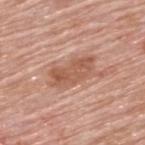Impression:
Imaged during a routine full-body skin examination; the lesion was not biopsied and no histopathology is available.
Background:
About 5.5 mm across. This is a white-light tile. A lesion tile, about 15 mm wide, cut from a 3D total-body photograph. Automated image analysis of the tile measured a footprint of about 11 mm² and an outline eccentricity of about 0.9 (0 = round, 1 = elongated). It also reported a classifier nevus-likeness of about 25/100 and lesion-presence confidence of about 100/100. The subject is a male about 80 years old. Located on the upper back.